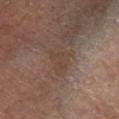Case summary:
• workup · catalogued during a skin exam; not biopsied
• site · the right lower leg
• patient · male, aged around 60
• acquisition · 15 mm crop, total-body photography